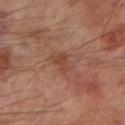Assessment: This lesion was catalogued during total-body skin photography and was not selected for biopsy. Context: About 2.5 mm across. The subject is a male approximately 70 years of age. This image is a 15 mm lesion crop taken from a total-body photograph. This is a cross-polarized tile. The lesion is on the left lower leg.